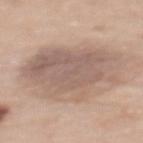Q: Is there a histopathology result?
A: no biopsy performed (imaged during a skin exam)
Q: Who is the patient?
A: female, aged 58 to 62
Q: Lesion location?
A: the upper back
Q: How was this image acquired?
A: ~15 mm tile from a whole-body skin photo
Q: Automated lesion metrics?
A: an average lesion color of about L≈59 a*≈16 b*≈25 (CIELAB), roughly 10 lightness units darker than nearby skin, and a lesion-to-skin contrast of about 6.5 (normalized; higher = more distinct); border irregularity of about 3.5 on a 0–10 scale, a color-variation rating of about 4.5/10, and radial color variation of about 1.5; a nevus-likeness score of about 5/100
Q: What lighting was used for the tile?
A: white-light illumination